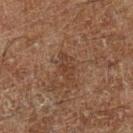Case summary:
• notes — total-body-photography surveillance lesion; no biopsy
• lesion size — ≈3 mm
• patient — male, aged around 60
• image source — ~15 mm crop, total-body skin-cancer survey
• TBP lesion metrics — an eccentricity of roughly 0.9 and a symmetry-axis asymmetry near 0.4
• anatomic site — the left lower leg